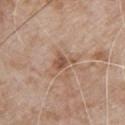– notes — imaged on a skin check; not biopsied
– image-analysis metrics — an area of roughly 3.5 mm², an eccentricity of roughly 0.75, and a shape-asymmetry score of about 0.45 (0 = symmetric); about 10 CIELAB-L* units darker than the surrounding skin and a normalized lesion–skin contrast near 7; border irregularity of about 4.5 on a 0–10 scale, a within-lesion color-variation index near 1.5/10, and a peripheral color-asymmetry measure near 0.5; a classifier nevus-likeness of about 0/100 and a lesion-detection confidence of about 100/100
– diameter — about 3 mm
– subject — male, aged 78 to 82
– acquisition — ~15 mm crop, total-body skin-cancer survey
– body site — the chest